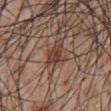• notes — total-body-photography surveillance lesion; no biopsy
• lesion diameter — ≈3 mm
• automated metrics — an average lesion color of about L≈40 a*≈18 b*≈25 (CIELAB), about 11 CIELAB-L* units darker than the surrounding skin, and a lesion-to-skin contrast of about 9 (normalized; higher = more distinct); a classifier nevus-likeness of about 20/100 and a detector confidence of about 100 out of 100 that the crop contains a lesion
• site — the abdomen
• imaging modality — total-body-photography crop, ~15 mm field of view
• subject — male, aged around 55